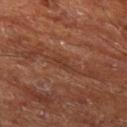<lesion>
  <patient>
    <sex>male</sex>
    <age_approx>65</age_approx>
  </patient>
  <site>left thigh</site>
  <lesion_size>
    <long_diameter_mm_approx>2.5</long_diameter_mm_approx>
  </lesion_size>
  <image>
    <source>total-body photography crop</source>
    <field_of_view_mm>15</field_of_view_mm>
  </image>
</lesion>